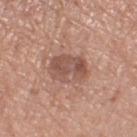Captured during whole-body skin photography for melanoma surveillance; the lesion was not biopsied.
Cropped from a total-body skin-imaging series; the visible field is about 15 mm.
The lesion is located on the right thigh.
The lesion's longest dimension is about 4 mm.
The subject is a female aged around 75.